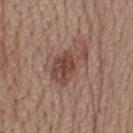Part of a total-body skin-imaging series; this lesion was reviewed on a skin check and was not flagged for biopsy. The tile uses white-light illumination. Cropped from a total-body skin-imaging series; the visible field is about 15 mm. The lesion's longest dimension is about 5.5 mm. The lesion is on the head or neck. A female patient, in their mid-50s.